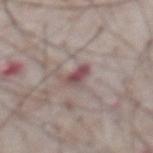| field | value |
|---|---|
| notes | imaged on a skin check; not biopsied |
| illumination | white-light |
| lesion diameter | about 2.5 mm |
| image-analysis metrics | a footprint of about 4 mm², a shape eccentricity near 0.75, and a symmetry-axis asymmetry near 0.35; an average lesion color of about L≈49 a*≈19 b*≈16 (CIELAB) and a lesion–skin lightness drop of about 11 |
| subject | male, about 75 years old |
| site | the abdomen |
| imaging modality | ~15 mm tile from a whole-body skin photo |